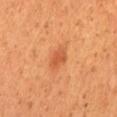The lesion was tiled from a total-body skin photograph and was not biopsied. The lesion is on the back. A lesion tile, about 15 mm wide, cut from a 3D total-body photograph. The patient is a male aged 43 to 47.